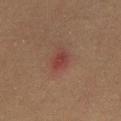follow-up — imaged on a skin check; not biopsied | diameter — about 3 mm | illumination — cross-polarized | subject — male, approximately 60 years of age | imaging modality — ~15 mm crop, total-body skin-cancer survey | image-analysis metrics — an outline eccentricity of about 0.8 (0 = round, 1 = elongated) and two-axis asymmetry of about 0.3; a border-irregularity index near 3/10, a color-variation rating of about 1.5/10, and a peripheral color-asymmetry measure near 0.5 | site — the chest.